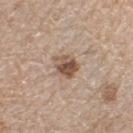Findings:
– follow-up · total-body-photography surveillance lesion; no biopsy
– patient · female, roughly 70 years of age
– site · the right lower leg
– acquisition · 15 mm crop, total-body photography